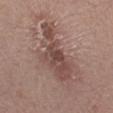The lesion is located on the left forearm. Measured at roughly 10 mm in maximum diameter. An algorithmic analysis of the crop reported about 10 CIELAB-L* units darker than the surrounding skin. And it measured a border-irregularity index near 7.5/10, internal color variation of about 4.5 on a 0–10 scale, and radial color variation of about 1. And it measured a nevus-likeness score of about 20/100 and a detector confidence of about 90 out of 100 that the crop contains a lesion. A 15 mm close-up tile from a total-body photography series done for melanoma screening. A female patient aged 28–32.